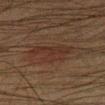{
  "biopsy_status": "not biopsied; imaged during a skin examination",
  "lighting": "cross-polarized",
  "automated_metrics": {
    "area_mm2_approx": 9.5,
    "vs_skin_darker_L": 4.0,
    "color_variation_0_10": 2.0,
    "peripheral_color_asymmetry": 1.0,
    "nevus_likeness_0_100": 15,
    "lesion_detection_confidence_0_100": 95
  },
  "image": {
    "source": "total-body photography crop",
    "field_of_view_mm": 15
  },
  "lesion_size": {
    "long_diameter_mm_approx": 5.0
  },
  "site": "left lower leg",
  "patient": {
    "sex": "male",
    "age_approx": 35
  }
}